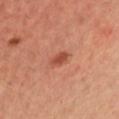{
  "biopsy_status": "not biopsied; imaged during a skin examination",
  "patient": {
    "sex": "male",
    "age_approx": 35
  },
  "site": "chest",
  "image": {
    "source": "total-body photography crop",
    "field_of_view_mm": 15
  }
}